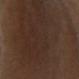Q: Was this lesion biopsied?
A: total-body-photography surveillance lesion; no biopsy
Q: Where on the body is the lesion?
A: the right upper arm
Q: How was this image acquired?
A: ~15 mm tile from a whole-body skin photo
Q: What are the patient's age and sex?
A: male, aged 73–77